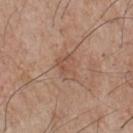The lesion was tiled from a total-body skin photograph and was not biopsied. Approximately 3 mm at its widest. A 15 mm close-up extracted from a 3D total-body photography capture. A male subject, approximately 75 years of age. The lesion is located on the front of the torso.